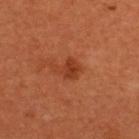| field | value |
|---|---|
| notes | imaged on a skin check; not biopsied |
| subject | female, aged approximately 50 |
| tile lighting | cross-polarized |
| diameter | ~2.5 mm (longest diameter) |
| image source | ~15 mm tile from a whole-body skin photo |